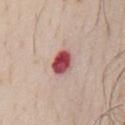follow-up: total-body-photography surveillance lesion; no biopsy | imaging modality: 15 mm crop, total-body photography | lesion diameter: ≈3.5 mm | subject: male, roughly 70 years of age | location: the chest.